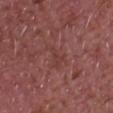Q: Was a biopsy performed?
A: imaged on a skin check; not biopsied
Q: Where on the body is the lesion?
A: the chest
Q: How was the tile lit?
A: white-light illumination
Q: What is the imaging modality?
A: ~15 mm crop, total-body skin-cancer survey
Q: What is the lesion's diameter?
A: ≈3.5 mm
Q: Who is the patient?
A: male, about 65 years old